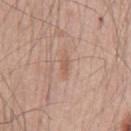No biopsy was performed on this lesion — it was imaged during a full skin examination and was not determined to be concerning.
Located on the chest.
The lesion-visualizer software estimated an eccentricity of roughly 0.95. It also reported a mean CIELAB color near L≈58 a*≈20 b*≈29, about 7 CIELAB-L* units darker than the surrounding skin, and a normalized lesion–skin contrast near 5.5. The software also gave lesion-presence confidence of about 100/100.
The recorded lesion diameter is about 2.5 mm.
The tile uses white-light illumination.
Cropped from a total-body skin-imaging series; the visible field is about 15 mm.
A male patient aged 53 to 57.